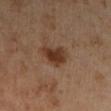The lesion was tiled from a total-body skin photograph and was not biopsied.
A 15 mm close-up extracted from a 3D total-body photography capture.
Captured under cross-polarized illumination.
A male patient, approximately 60 years of age.
On the left lower leg.
The total-body-photography lesion software estimated an area of roughly 6.5 mm², an outline eccentricity of about 0.6 (0 = round, 1 = elongated), and two-axis asymmetry of about 0.35. The software also gave a border-irregularity rating of about 3.5/10 and a peripheral color-asymmetry measure near 0.5. The analysis additionally found a nevus-likeness score of about 95/100 and a detector confidence of about 100 out of 100 that the crop contains a lesion.
The lesion's longest dimension is about 3.5 mm.